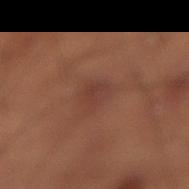Assessment: Part of a total-body skin-imaging series; this lesion was reviewed on a skin check and was not flagged for biopsy. Context: A 15 mm close-up extracted from a 3D total-body photography capture. A male subject, roughly 50 years of age. The total-body-photography lesion software estimated an average lesion color of about L≈29 a*≈18 b*≈20 (CIELAB), about 4 CIELAB-L* units darker than the surrounding skin, and a lesion-to-skin contrast of about 5 (normalized; higher = more distinct). The software also gave border irregularity of about 2.5 on a 0–10 scale and a within-lesion color-variation index near 2/10. Captured under cross-polarized illumination. The lesion's longest dimension is about 3 mm. On the lower back.